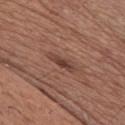notes: imaged on a skin check; not biopsied
imaging modality: total-body-photography crop, ~15 mm field of view
patient: male, aged 48–52
body site: the chest